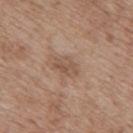{
  "site": "mid back",
  "image": {
    "source": "total-body photography crop",
    "field_of_view_mm": 15
  },
  "patient": {
    "sex": "male",
    "age_approx": 70
  }
}